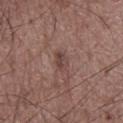Captured during whole-body skin photography for melanoma surveillance; the lesion was not biopsied.